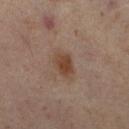Captured during whole-body skin photography for melanoma surveillance; the lesion was not biopsied.
A female subject approximately 40 years of age.
On the right lower leg.
A region of skin cropped from a whole-body photographic capture, roughly 15 mm wide.